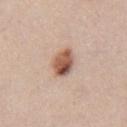Q: Was a biopsy performed?
A: catalogued during a skin exam; not biopsied
Q: What is the anatomic site?
A: the mid back
Q: What is the lesion's diameter?
A: ~4 mm (longest diameter)
Q: Illumination type?
A: white-light illumination
Q: What kind of image is this?
A: ~15 mm tile from a whole-body skin photo
Q: What are the patient's age and sex?
A: male, aged around 40